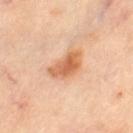<record>
  <biopsy_status>not biopsied; imaged during a skin examination</biopsy_status>
  <image>
    <source>total-body photography crop</source>
    <field_of_view_mm>15</field_of_view_mm>
  </image>
  <lesion_size>
    <long_diameter_mm_approx>4.0</long_diameter_mm_approx>
  </lesion_size>
  <automated_metrics>
    <eccentricity>0.75</eccentricity>
    <shape_asymmetry>0.3</shape_asymmetry>
    <cielab_L>65</cielab_L>
    <cielab_a>26</cielab_a>
    <cielab_b>39</cielab_b>
    <vs_skin_darker_L>12.0</vs_skin_darker_L>
    <vs_skin_contrast_norm>8.0</vs_skin_contrast_norm>
    <border_irregularity_0_10>3.0</border_irregularity_0_10>
    <color_variation_0_10>4.5</color_variation_0_10>
    <peripheral_color_asymmetry>1.5</peripheral_color_asymmetry>
  </automated_metrics>
  <patient>
    <sex>female</sex>
    <age_approx>60</age_approx>
  </patient>
  <site>right leg</site>
  <lighting>cross-polarized</lighting>
</record>